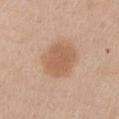<tbp_lesion>
<site>left upper arm</site>
<image>
  <source>total-body photography crop</source>
  <field_of_view_mm>15</field_of_view_mm>
</image>
<lighting>white-light</lighting>
<patient>
  <sex>male</sex>
  <age_approx>60</age_approx>
</patient>
<lesion_size>
  <long_diameter_mm_approx>5.0</long_diameter_mm_approx>
</lesion_size>
</tbp_lesion>